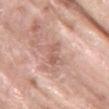The lesion was photographed on a routine skin check and not biopsied; there is no pathology result. A close-up tile cropped from a whole-body skin photograph, about 15 mm across. Captured under white-light illumination. The patient is a female aged 68 to 72. Measured at roughly 3.5 mm in maximum diameter. Located on the upper back. Automated tile analysis of the lesion measured an area of roughly 5.5 mm², an eccentricity of roughly 0.8, and a shape-asymmetry score of about 0.4 (0 = symmetric). The analysis additionally found a border-irregularity index near 4.5/10, a color-variation rating of about 2/10, and a peripheral color-asymmetry measure near 0.5.